A male subject aged approximately 55 · a lesion tile, about 15 mm wide, cut from a 3D total-body photograph · the lesion is on the chest:
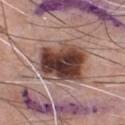lesion diameter: ≈6 mm; illumination: white-light illumination; pathology: a dysplastic (Clark) nevus.